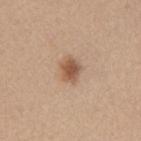Clinical impression: Recorded during total-body skin imaging; not selected for excision or biopsy. Clinical summary: Cropped from a total-body skin-imaging series; the visible field is about 15 mm. On the mid back. A female patient, in their 40s.